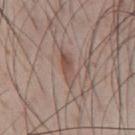Q: Was this lesion biopsied?
A: total-body-photography surveillance lesion; no biopsy
Q: What is the imaging modality?
A: 15 mm crop, total-body photography
Q: What is the lesion's diameter?
A: ≈4 mm
Q: Patient demographics?
A: male, aged around 55
Q: Illumination type?
A: white-light illumination
Q: What is the anatomic site?
A: the front of the torso